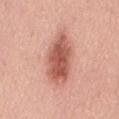Q: Is there a histopathology result?
A: total-body-photography surveillance lesion; no biopsy
Q: Patient demographics?
A: male, aged 53–57
Q: Automated lesion metrics?
A: a footprint of about 17 mm² and an eccentricity of roughly 0.9; a border-irregularity index near 2.5/10, a within-lesion color-variation index near 5/10, and a peripheral color-asymmetry measure near 1.5; an automated nevus-likeness rating near 95 out of 100 and a detector confidence of about 100 out of 100 that the crop contains a lesion
Q: What kind of image is this?
A: ~15 mm crop, total-body skin-cancer survey
Q: Lesion location?
A: the mid back
Q: Lesion size?
A: ~7 mm (longest diameter)